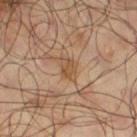workup: total-body-photography surveillance lesion; no biopsy | body site: the right thigh | acquisition: ~15 mm crop, total-body skin-cancer survey | subject: male, aged 63–67 | size: about 3 mm.